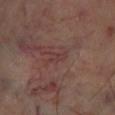biopsy_status: not biopsied; imaged during a skin examination
lesion_size:
  long_diameter_mm_approx: 2.5
image:
  source: total-body photography crop
  field_of_view_mm: 15
automated_metrics:
  area_mm2_approx: 3.0
  eccentricity: 0.85
  shape_asymmetry: 0.3
site: leg